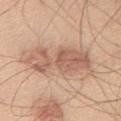biopsy status = imaged on a skin check; not biopsied
patient = male, aged around 45
body site = the chest
TBP lesion metrics = a border-irregularity index near 5/10, a color-variation rating of about 4.5/10, and radial color variation of about 1.5
imaging modality = 15 mm crop, total-body photography